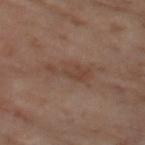Impression: Captured during whole-body skin photography for melanoma surveillance; the lesion was not biopsied. Background: Cropped from a whole-body photographic skin survey; the tile spans about 15 mm. A female subject, aged 53–57. On the left thigh.